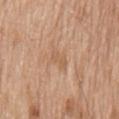lighting: white-light; patient: male, aged approximately 80; lesion diameter: about 3 mm; acquisition: ~15 mm crop, total-body skin-cancer survey; location: the mid back; automated metrics: a mean CIELAB color near L≈59 a*≈19 b*≈33, a lesion–skin lightness drop of about 6, and a normalized lesion–skin contrast near 4.5.